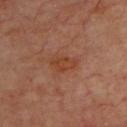<tbp_lesion>
  <biopsy_status>not biopsied; imaged during a skin examination</biopsy_status>
  <lesion_size>
    <long_diameter_mm_approx>3.5</long_diameter_mm_approx>
  </lesion_size>
  <site>back</site>
  <image>
    <source>total-body photography crop</source>
    <field_of_view_mm>15</field_of_view_mm>
  </image>
  <patient>
    <sex>male</sex>
    <age_approx>60</age_approx>
  </patient>
</tbp_lesion>